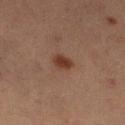biopsy_status: not biopsied; imaged during a skin examination
site: left lower leg
patient:
  sex: female
  age_approx: 55
image:
  source: total-body photography crop
  field_of_view_mm: 15